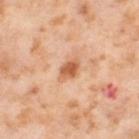Impression:
Recorded during total-body skin imaging; not selected for excision or biopsy.
Acquisition and patient details:
The lesion is on the leg. A 15 mm close-up extracted from a 3D total-body photography capture. A female patient, roughly 55 years of age.